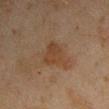Part of a total-body skin-imaging series; this lesion was reviewed on a skin check and was not flagged for biopsy.
From the right upper arm.
A male subject about 45 years old.
The tile uses cross-polarized illumination.
A region of skin cropped from a whole-body photographic capture, roughly 15 mm wide.
Longest diameter approximately 4.5 mm.